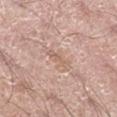  biopsy_status: not biopsied; imaged during a skin examination
  automated_metrics:
    cielab_L: 62
    cielab_a: 18
    cielab_b: 27
    vs_skin_darker_L: 7.0
    vs_skin_contrast_norm: 4.5
    border_irregularity_0_10: 4.0
    peripheral_color_asymmetry: 0.5
    nevus_likeness_0_100: 0
    lesion_detection_confidence_0_100: 95
  patient:
    sex: male
    age_approx: 60
  lighting: white-light
  lesion_size:
    long_diameter_mm_approx: 3.0
  image:
    source: total-body photography crop
    field_of_view_mm: 15
  site: right lower leg